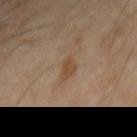Captured during whole-body skin photography for melanoma surveillance; the lesion was not biopsied.
From the mid back.
About 3 mm across.
Cropped from a total-body skin-imaging series; the visible field is about 15 mm.
Captured under cross-polarized illumination.
The total-body-photography lesion software estimated an area of roughly 3.5 mm², an outline eccentricity of about 0.85 (0 = round, 1 = elongated), and a shape-asymmetry score of about 0.3 (0 = symmetric). The software also gave a mean CIELAB color near L≈45 a*≈16 b*≈31 and a normalized lesion–skin contrast near 7. The software also gave a classifier nevus-likeness of about 10/100 and a lesion-detection confidence of about 100/100.
A male subject roughly 60 years of age.